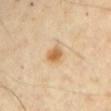Recorded during total-body skin imaging; not selected for excision or biopsy. Located on the left upper arm. Cropped from a whole-body photographic skin survey; the tile spans about 15 mm. An algorithmic analysis of the crop reported two-axis asymmetry of about 0.25. The analysis additionally found a mean CIELAB color near L≈63 a*≈18 b*≈40 and about 11 CIELAB-L* units darker than the surrounding skin. The analysis additionally found a border-irregularity index near 2/10 and a within-lesion color-variation index near 3.5/10. And it measured a nevus-likeness score of about 95/100. The patient is a male aged around 65. Measured at roughly 2.5 mm in maximum diameter.